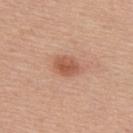follow-up = imaged on a skin check; not biopsied | subject = male, about 55 years old | tile lighting = white-light illumination | anatomic site = the back | image-analysis metrics = a lesion color around L≈55 a*≈25 b*≈32 in CIELAB, roughly 11 lightness units darker than nearby skin, and a normalized border contrast of about 7.5; border irregularity of about 1.5 on a 0–10 scale, a within-lesion color-variation index near 3/10, and radial color variation of about 1 | image = total-body-photography crop, ~15 mm field of view | lesion size = about 3 mm.